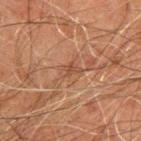imaging modality: ~15 mm crop, total-body skin-cancer survey
body site: the upper back
patient: male, about 45 years old
diameter: ~2.5 mm (longest diameter)
image-analysis metrics: a footprint of about 3.5 mm²; an average lesion color of about L≈41 a*≈19 b*≈27 (CIELAB), a lesion–skin lightness drop of about 6, and a lesion-to-skin contrast of about 5.5 (normalized; higher = more distinct)
illumination: cross-polarized illumination